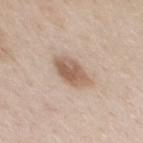Recorded during total-body skin imaging; not selected for excision or biopsy.
A region of skin cropped from a whole-body photographic capture, roughly 15 mm wide.
On the chest.
Longest diameter approximately 4 mm.
The patient is a male roughly 60 years of age.
Imaged with white-light lighting.